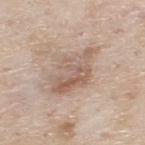acquisition = 15 mm crop, total-body photography; anatomic site = the upper back; automated lesion analysis = an average lesion color of about L≈59 a*≈16 b*≈26 (CIELAB) and roughly 9 lightness units darker than nearby skin; lesion size = ~6.5 mm (longest diameter); tile lighting = white-light illumination; patient = male, aged around 80.